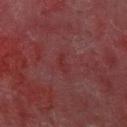Q: Was a biopsy performed?
A: total-body-photography surveillance lesion; no biopsy
Q: Who is the patient?
A: male, in their mid-50s
Q: What did automated image analysis measure?
A: a mean CIELAB color near L≈28 a*≈27 b*≈19, about 4 CIELAB-L* units darker than the surrounding skin, and a normalized lesion–skin contrast near 5; border irregularity of about 4.5 on a 0–10 scale, a within-lesion color-variation index near 0/10, and radial color variation of about 0; lesion-presence confidence of about 75/100
Q: Lesion size?
A: about 2.5 mm
Q: What is the imaging modality?
A: ~15 mm crop, total-body skin-cancer survey
Q: How was the tile lit?
A: cross-polarized
Q: What is the anatomic site?
A: the right thigh